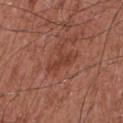Case summary:
• notes · catalogued during a skin exam; not biopsied